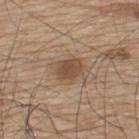Impression: Recorded during total-body skin imaging; not selected for excision or biopsy. Acquisition and patient details: The tile uses white-light illumination. About 3.5 mm across. A male subject, in their mid- to late 70s. From the upper back. A close-up tile cropped from a whole-body skin photograph, about 15 mm across.